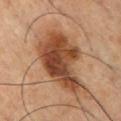Captured during whole-body skin photography for melanoma surveillance; the lesion was not biopsied. A male patient aged around 55. Cropped from a whole-body photographic skin survey; the tile spans about 15 mm. The lesion is located on the front of the torso.